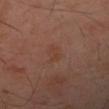workup: imaged on a skin check; not biopsied
automated lesion analysis: a lesion area of about 3.5 mm², an outline eccentricity of about 0.75 (0 = round, 1 = elongated), and a shape-asymmetry score of about 0.3 (0 = symmetric); a mean CIELAB color near L≈40 a*≈21 b*≈28, about 4 CIELAB-L* units darker than the surrounding skin, and a normalized lesion–skin contrast near 5; border irregularity of about 3.5 on a 0–10 scale, internal color variation of about 2.5 on a 0–10 scale, and a peripheral color-asymmetry measure near 1; an automated nevus-likeness rating near 0 out of 100 and lesion-presence confidence of about 100/100
subject: female, about 55 years old
site: the arm
diameter: ~2.5 mm (longest diameter)
lighting: cross-polarized
image: ~15 mm tile from a whole-body skin photo